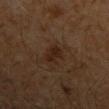follow-up: catalogued during a skin exam; not biopsied | diameter: ~3 mm (longest diameter) | acquisition: ~15 mm tile from a whole-body skin photo | subject: male, roughly 60 years of age | anatomic site: the arm.